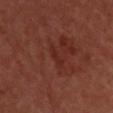| field | value |
|---|---|
| follow-up | total-body-photography surveillance lesion; no biopsy |
| body site | the back |
| patient | male, approximately 50 years of age |
| image source | 15 mm crop, total-body photography |
| tile lighting | cross-polarized illumination |
| size | ~2.5 mm (longest diameter) |